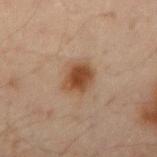The lesion was tiled from a total-body skin photograph and was not biopsied.
The patient is a male approximately 50 years of age.
A 15 mm close-up tile from a total-body photography series done for melanoma screening.
About 3.5 mm across.
Automated tile analysis of the lesion measured a lesion area of about 8 mm² and a shape-asymmetry score of about 0.2 (0 = symmetric). The software also gave a lesion color around L≈42 a*≈19 b*≈30 in CIELAB and a lesion–skin lightness drop of about 11. It also reported a border-irregularity index near 2/10, a color-variation rating of about 3.5/10, and a peripheral color-asymmetry measure near 1. And it measured a classifier nevus-likeness of about 100/100 and a lesion-detection confidence of about 100/100.
On the arm.